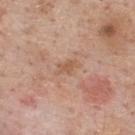{"image": {"source": "total-body photography crop", "field_of_view_mm": 15}, "site": "upper back", "lesion_size": {"long_diameter_mm_approx": 3.0}, "patient": {"sex": "male", "age_approx": 60}, "lighting": "white-light"}